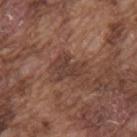Case summary:
* notes: catalogued during a skin exam; not biopsied
* site: the upper back
* subject: male, in their mid- to late 70s
* lesion diameter: ≈3.5 mm
* acquisition: 15 mm crop, total-body photography